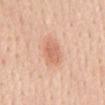notes — catalogued during a skin exam; not biopsied | body site — the mid back | imaging modality — ~15 mm tile from a whole-body skin photo | patient — female, aged around 45 | illumination — white-light.